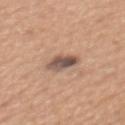Q: Is there a histopathology result?
A: no biopsy performed (imaged during a skin exam)
Q: Automated lesion metrics?
A: an area of roughly 6 mm² and two-axis asymmetry of about 0.25; a border-irregularity rating of about 2.5/10, internal color variation of about 7.5 on a 0–10 scale, and a peripheral color-asymmetry measure near 2.5; a nevus-likeness score of about 90/100 and a detector confidence of about 100 out of 100 that the crop contains a lesion
Q: What is the imaging modality?
A: 15 mm crop, total-body photography
Q: What are the patient's age and sex?
A: male, aged 53 to 57
Q: Where on the body is the lesion?
A: the mid back
Q: Illumination type?
A: white-light illumination